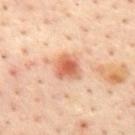<tbp_lesion>
<biopsy_status>not biopsied; imaged during a skin examination</biopsy_status>
<site>mid back</site>
<automated_metrics>
  <area_mm2_approx>7.0</area_mm2_approx>
  <eccentricity>0.35</eccentricity>
  <shape_asymmetry>0.2</shape_asymmetry>
  <cielab_L>62</cielab_L>
  <cielab_a>27</cielab_a>
  <cielab_b>35</cielab_b>
  <vs_skin_darker_L>13.0</vs_skin_darker_L>
  <border_irregularity_0_10>2.0</border_irregularity_0_10>
  <color_variation_0_10>5.0</color_variation_0_10>
  <peripheral_color_asymmetry>1.5</peripheral_color_asymmetry>
  <nevus_likeness_0_100>95</nevus_likeness_0_100>
</automated_metrics>
<patient>
  <sex>male</sex>
  <age_approx>45</age_approx>
</patient>
<image>
  <source>total-body photography crop</source>
  <field_of_view_mm>15</field_of_view_mm>
</image>
<lighting>cross-polarized</lighting>
</tbp_lesion>